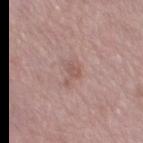This lesion was catalogued during total-body skin photography and was not selected for biopsy. A 15 mm crop from a total-body photograph taken for skin-cancer surveillance. A female subject, aged around 70. On the left thigh. This is a white-light tile. The recorded lesion diameter is about 3 mm.